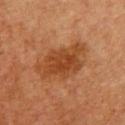Q: Was a biopsy performed?
A: no biopsy performed (imaged during a skin exam)
Q: How large is the lesion?
A: about 6.5 mm
Q: Lesion location?
A: the upper back
Q: Who is the patient?
A: male, aged 58–62
Q: What lighting was used for the tile?
A: cross-polarized illumination
Q: How was this image acquired?
A: ~15 mm tile from a whole-body skin photo